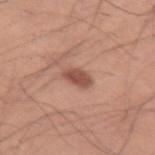Case summary:
• follow-up — imaged on a skin check; not biopsied
• image-analysis metrics — a lesion area of about 4 mm², an eccentricity of roughly 0.8, and a symmetry-axis asymmetry near 0.2; an average lesion color of about L≈51 a*≈24 b*≈28 (CIELAB), about 12 CIELAB-L* units darker than the surrounding skin, and a lesion-to-skin contrast of about 8.5 (normalized; higher = more distinct); a nevus-likeness score of about 90/100 and a lesion-detection confidence of about 100/100
• site — the left upper arm
• patient — male, aged 23 to 27
• acquisition — ~15 mm tile from a whole-body skin photo